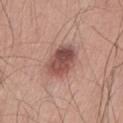biopsy status: total-body-photography surveillance lesion; no biopsy | image: total-body-photography crop, ~15 mm field of view | TBP lesion metrics: a footprint of about 12 mm², an outline eccentricity of about 0.75 (0 = round, 1 = elongated), and two-axis asymmetry of about 0.15; a border-irregularity rating of about 2/10, a within-lesion color-variation index near 6/10, and radial color variation of about 2; lesion-presence confidence of about 100/100 | patient: male, aged 48 to 52 | lesion diameter: ≈4.5 mm | body site: the left thigh.